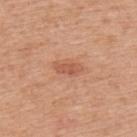Imaged during a routine full-body skin examination; the lesion was not biopsied and no histopathology is available.
Approximately 3 mm at its widest.
Located on the upper back.
Automated image analysis of the tile measured an average lesion color of about L≈56 a*≈26 b*≈33 (CIELAB), a lesion–skin lightness drop of about 9, and a normalized border contrast of about 6.5. And it measured a nevus-likeness score of about 35/100.
The subject is a male in their 50s.
A roughly 15 mm field-of-view crop from a total-body skin photograph.
Imaged with white-light lighting.